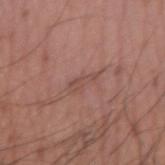Clinical impression: Imaged during a routine full-body skin examination; the lesion was not biopsied and no histopathology is available. Acquisition and patient details: The lesion's longest dimension is about 3 mm. The total-body-photography lesion software estimated a mean CIELAB color near L≈48 a*≈21 b*≈24, about 6 CIELAB-L* units darker than the surrounding skin, and a lesion-to-skin contrast of about 5 (normalized; higher = more distinct). The analysis additionally found a peripheral color-asymmetry measure near 0. A male patient, aged 48 to 52. Captured under white-light illumination. A 15 mm close-up tile from a total-body photography series done for melanoma screening. On the arm.